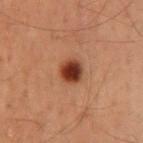workup = imaged on a skin check; not biopsied | lesion size = ≈2.5 mm | site = the lower back | patient = male, aged 58 to 62 | imaging modality = total-body-photography crop, ~15 mm field of view | lighting = cross-polarized.